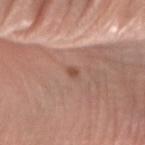{"biopsy_status": "not biopsied; imaged during a skin examination", "image": {"source": "total-body photography crop", "field_of_view_mm": 15}, "lesion_size": {"long_diameter_mm_approx": 1.5}, "patient": {"sex": "female", "age_approx": 50}, "site": "right forearm", "lighting": "white-light"}